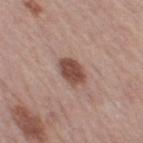The lesion was photographed on a routine skin check and not biopsied; there is no pathology result. A 15 mm crop from a total-body photograph taken for skin-cancer surveillance. Imaged with white-light lighting. The recorded lesion diameter is about 3.5 mm. A female subject, roughly 65 years of age. The lesion is on the leg. Automated image analysis of the tile measured a lesion area of about 7 mm², an eccentricity of roughly 0.7, and two-axis asymmetry of about 0.2. The analysis additionally found roughly 14 lightness units darker than nearby skin and a normalized lesion–skin contrast near 10. The analysis additionally found border irregularity of about 2 on a 0–10 scale and a color-variation rating of about 2/10.